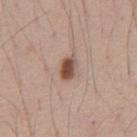• workup · catalogued during a skin exam; not biopsied
• patient · male, approximately 40 years of age
• size · about 2.5 mm
• automated metrics · an automated nevus-likeness rating near 100 out of 100 and a lesion-detection confidence of about 100/100
• site · the abdomen
• image source · ~15 mm crop, total-body skin-cancer survey